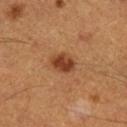biopsy_status: not biopsied; imaged during a skin examination
image:
  source: total-body photography crop
  field_of_view_mm: 15
patient:
  sex: male
  age_approx: 60
lighting: cross-polarized
site: right lower leg
lesion_size:
  long_diameter_mm_approx: 3.0
automated_metrics:
  area_mm2_approx: 6.0
  eccentricity: 0.65
  border_irregularity_0_10: 1.5
  color_variation_0_10: 2.5